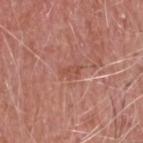<record>
  <biopsy_status>not biopsied; imaged during a skin examination</biopsy_status>
  <lesion_size>
    <long_diameter_mm_approx>2.5</long_diameter_mm_approx>
  </lesion_size>
  <image>
    <source>total-body photography crop</source>
    <field_of_view_mm>15</field_of_view_mm>
  </image>
  <lighting>white-light</lighting>
  <patient>
    <sex>male</sex>
    <age_approx>65</age_approx>
  </patient>
  <site>head or neck</site>
</record>